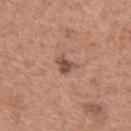Imaged during a routine full-body skin examination; the lesion was not biopsied and no histopathology is available. Automated tile analysis of the lesion measured border irregularity of about 3.5 on a 0–10 scale, a color-variation rating of about 1.5/10, and peripheral color asymmetry of about 0.5. And it measured a classifier nevus-likeness of about 65/100. Cropped from a whole-body photographic skin survey; the tile spans about 15 mm. The lesion is on the upper back. The patient is a female approximately 60 years of age. Captured under white-light illumination. The recorded lesion diameter is about 2.5 mm.